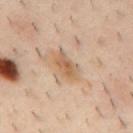Recorded during total-body skin imaging; not selected for excision or biopsy. A region of skin cropped from a whole-body photographic capture, roughly 15 mm wide. A male patient about 40 years old. Automated tile analysis of the lesion measured an area of roughly 6 mm² and two-axis asymmetry of about 0.25. And it measured a normalized border contrast of about 7. It also reported a nevus-likeness score of about 0/100 and a detector confidence of about 95 out of 100 that the crop contains a lesion. The lesion is on the mid back. The tile uses cross-polarized illumination. About 4 mm across.